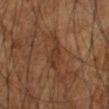{"biopsy_status": "not biopsied; imaged during a skin examination", "image": {"source": "total-body photography crop", "field_of_view_mm": 15}, "site": "left arm", "lighting": "cross-polarized", "patient": {"sex": "male", "age_approx": 65}, "automated_metrics": {"area_mm2_approx": 5.5, "eccentricity": 0.9, "shape_asymmetry": 0.4, "border_irregularity_0_10": 4.5, "peripheral_color_asymmetry": 0.5}}